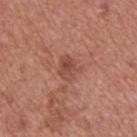Q: Was this lesion biopsied?
A: catalogued during a skin exam; not biopsied
Q: What is the lesion's diameter?
A: ~2.5 mm (longest diameter)
Q: What is the imaging modality?
A: ~15 mm crop, total-body skin-cancer survey
Q: Automated lesion metrics?
A: a lesion area of about 5.5 mm² and a shape-asymmetry score of about 0.3 (0 = symmetric)
Q: What are the patient's age and sex?
A: male, aged 68 to 72
Q: Where on the body is the lesion?
A: the upper back